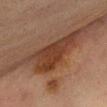Recorded during total-body skin imaging; not selected for excision or biopsy. The lesion is on the front of the torso. The lesion's longest dimension is about 7 mm. A female patient, approximately 65 years of age. Automated image analysis of the tile measured border irregularity of about 5 on a 0–10 scale, a color-variation rating of about 4.5/10, and peripheral color asymmetry of about 1.5. A 15 mm close-up tile from a total-body photography series done for melanoma screening.